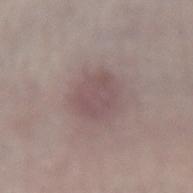| feature | finding |
|---|---|
| biopsy status | imaged on a skin check; not biopsied |
| patient | female, aged approximately 50 |
| anatomic site | the leg |
| imaging modality | total-body-photography crop, ~15 mm field of view |
| automated metrics | a footprint of about 11 mm², an eccentricity of roughly 0.55, and a shape-asymmetry score of about 0.15 (0 = symmetric); a mean CIELAB color near L≈50 a*≈16 b*≈16, a lesion–skin lightness drop of about 7, and a lesion-to-skin contrast of about 5.5 (normalized; higher = more distinct); an automated nevus-likeness rating near 5 out of 100 |
| tile lighting | white-light illumination |